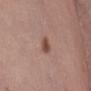notes = no biopsy performed (imaged during a skin exam)
automated metrics = an average lesion color of about L≈49 a*≈21 b*≈27 (CIELAB), a lesion–skin lightness drop of about 10, and a normalized lesion–skin contrast near 8; border irregularity of about 2.5 on a 0–10 scale, a color-variation rating of about 2.5/10, and a peripheral color-asymmetry measure near 1
illumination = white-light illumination
lesion size = ≈2.5 mm
patient = female, aged approximately 55
site = the abdomen
acquisition = ~15 mm crop, total-body skin-cancer survey